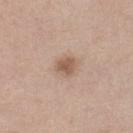follow-up — total-body-photography surveillance lesion; no biopsy
imaging modality — ~15 mm tile from a whole-body skin photo
location — the leg
diameter — ≈2.5 mm
subject — female, in their mid-50s